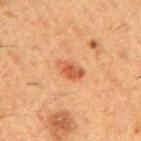Recorded during total-body skin imaging; not selected for excision or biopsy.
A 15 mm close-up extracted from a 3D total-body photography capture.
On the right upper arm.
The lesion-visualizer software estimated an area of roughly 5.5 mm², a shape eccentricity near 0.8, and two-axis asymmetry of about 0.25.
Approximately 3.5 mm at its widest.
Imaged with cross-polarized lighting.
A male patient, in their 50s.